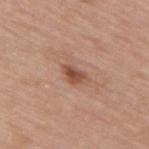* follow-up · no biopsy performed (imaged during a skin exam)
* lighting · white-light illumination
* automated lesion analysis · a footprint of about 4 mm², an eccentricity of roughly 0.8, and a symmetry-axis asymmetry near 0.4; internal color variation of about 4 on a 0–10 scale
* lesion diameter · about 3 mm
* acquisition · 15 mm crop, total-body photography
* location · the back
* subject · male, about 55 years old